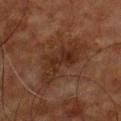The lesion was photographed on a routine skin check and not biopsied; there is no pathology result. This is a cross-polarized tile. The subject is a male in their 60s. The lesion is located on the chest. Approximately 7 mm at its widest. Automated image analysis of the tile measured a lesion area of about 15 mm², a shape eccentricity near 0.9, and two-axis asymmetry of about 0.45. And it measured border irregularity of about 6 on a 0–10 scale, internal color variation of about 3.5 on a 0–10 scale, and peripheral color asymmetry of about 1. A lesion tile, about 15 mm wide, cut from a 3D total-body photograph.